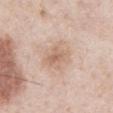Q: Is there a histopathology result?
A: no biopsy performed (imaged during a skin exam)
Q: What did automated image analysis measure?
A: a mean CIELAB color near L≈66 a*≈17 b*≈29, about 8 CIELAB-L* units darker than the surrounding skin, and a normalized border contrast of about 5.5
Q: What lighting was used for the tile?
A: white-light
Q: Where on the body is the lesion?
A: the chest
Q: What is the lesion's diameter?
A: ~5 mm (longest diameter)
Q: What kind of image is this?
A: 15 mm crop, total-body photography
Q: What are the patient's age and sex?
A: male, aged 53–57